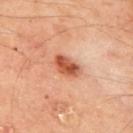follow-up: no biopsy performed (imaged during a skin exam) | image source: ~15 mm crop, total-body skin-cancer survey | diameter: ~3.5 mm (longest diameter) | location: the upper back | patient: male, approximately 65 years of age.